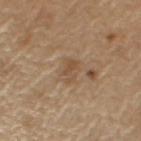| feature | finding |
|---|---|
| location | the left upper arm |
| acquisition | ~15 mm crop, total-body skin-cancer survey |
| subject | male, in their 70s |
| lesion size | ≈3 mm |
| image-analysis metrics | a mean CIELAB color near L≈50 a*≈16 b*≈32 and about 7 CIELAB-L* units darker than the surrounding skin; border irregularity of about 4 on a 0–10 scale and a color-variation rating of about 1/10 |
| tile lighting | white-light illumination |